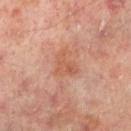The lesion was tiled from a total-body skin photograph and was not biopsied.
A male subject, about 65 years old.
The lesion is located on the left lower leg.
Cropped from a total-body skin-imaging series; the visible field is about 15 mm.
The total-body-photography lesion software estimated an area of roughly 6 mm² and two-axis asymmetry of about 0.45. It also reported internal color variation of about 3 on a 0–10 scale and a peripheral color-asymmetry measure near 1.5. It also reported a classifier nevus-likeness of about 0/100 and a lesion-detection confidence of about 100/100.
The tile uses cross-polarized illumination.